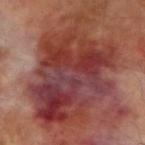Impression:
Recorded during total-body skin imaging; not selected for excision or biopsy.
Clinical summary:
A 15 mm close-up extracted from a 3D total-body photography capture. Captured under cross-polarized illumination. On the right upper arm. Measured at roughly 14 mm in maximum diameter. A male subject, about 70 years old. Automated image analysis of the tile measured an area of roughly 80 mm², an outline eccentricity of about 0.75 (0 = round, 1 = elongated), and a symmetry-axis asymmetry near 0.3. The analysis additionally found an average lesion color of about L≈38 a*≈27 b*≈24 (CIELAB) and roughly 12 lightness units darker than nearby skin.